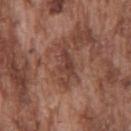biopsy status: imaged on a skin check; not biopsied
site: the chest
lighting: white-light
image: total-body-photography crop, ~15 mm field of view
automated lesion analysis: a lesion color around L≈42 a*≈21 b*≈25 in CIELAB and about 8 CIELAB-L* units darker than the surrounding skin; a color-variation rating of about 3/10 and a peripheral color-asymmetry measure near 1; a detector confidence of about 55 out of 100 that the crop contains a lesion
lesion size: ≈6 mm
patient: male, aged around 75